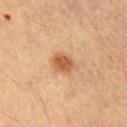The lesion is on the left upper arm. A 15 mm close-up tile from a total-body photography series done for melanoma screening. Imaged with cross-polarized lighting. Approximately 3 mm at its widest. A male subject, aged approximately 55.